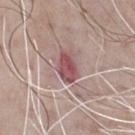– biopsy status · catalogued during a skin exam; not biopsied
– acquisition · total-body-photography crop, ~15 mm field of view
– subject · male, aged around 70
– body site · the chest
– illumination · white-light